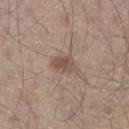The lesion was tiled from a total-body skin photograph and was not biopsied. Imaged with white-light lighting. Located on the right lower leg. A close-up tile cropped from a whole-body skin photograph, about 15 mm across. Approximately 2.5 mm at its widest. The patient is a male about 55 years old.